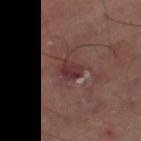Q: How was the tile lit?
A: cross-polarized illumination
Q: How large is the lesion?
A: ≈2.5 mm
Q: Automated lesion metrics?
A: a lesion area of about 5.5 mm² and an outline eccentricity of about 0.25 (0 = round, 1 = elongated)
Q: Lesion location?
A: the left thigh
Q: What kind of image is this?
A: 15 mm crop, total-body photography